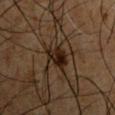follow-up: total-body-photography surveillance lesion; no biopsy
anatomic site: the left upper arm
patient: male, about 50 years old
lesion size: ≈3 mm
image: ~15 mm tile from a whole-body skin photo
TBP lesion metrics: an outline eccentricity of about 0.6 (0 = round, 1 = elongated) and a symmetry-axis asymmetry near 0.3; a normalized lesion–skin contrast near 12; a border-irregularity index near 3/10 and internal color variation of about 5.5 on a 0–10 scale; an automated nevus-likeness rating near 70 out of 100 and a lesion-detection confidence of about 90/100